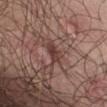follow-up = total-body-photography surveillance lesion; no biopsy | subject = male, roughly 50 years of age | body site = the chest | imaging modality = ~15 mm tile from a whole-body skin photo.